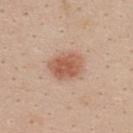A region of skin cropped from a whole-body photographic capture, roughly 15 mm wide. On the upper back. A female subject approximately 25 years of age. The recorded lesion diameter is about 4 mm.